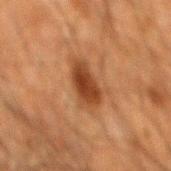Imaged during a routine full-body skin examination; the lesion was not biopsied and no histopathology is available.
The recorded lesion diameter is about 5 mm.
A male subject in their 60s.
This is a cross-polarized tile.
From the mid back.
A 15 mm close-up tile from a total-body photography series done for melanoma screening.